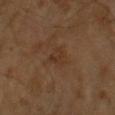Q: Is there a histopathology result?
A: imaged on a skin check; not biopsied
Q: What is the lesion's diameter?
A: ≈2.5 mm
Q: What are the patient's age and sex?
A: male, in their mid- to late 60s
Q: What lighting was used for the tile?
A: cross-polarized illumination
Q: How was this image acquired?
A: total-body-photography crop, ~15 mm field of view